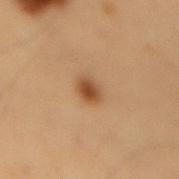Findings:
• notes: total-body-photography surveillance lesion; no biopsy
• anatomic site: the lower back
• subject: male, aged around 55
• image: ~15 mm crop, total-body skin-cancer survey